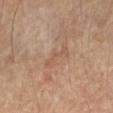site = the right forearm
automated metrics = a footprint of about 3 mm² and two-axis asymmetry of about 0.5; a nevus-likeness score of about 0/100 and lesion-presence confidence of about 95/100
size = ≈3.5 mm
lighting = cross-polarized illumination
image = ~15 mm tile from a whole-body skin photo
subject = female, aged 63 to 67